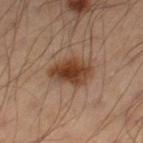notes: total-body-photography surveillance lesion; no biopsy | lesion size: about 4.5 mm | location: the left leg | patient: male, aged 48 to 52 | image: 15 mm crop, total-body photography.